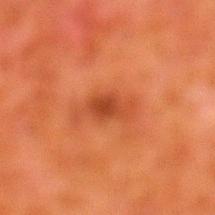Q: Is there a histopathology result?
A: catalogued during a skin exam; not biopsied
Q: Illumination type?
A: cross-polarized illumination
Q: What is the anatomic site?
A: the leg
Q: How was this image acquired?
A: 15 mm crop, total-body photography
Q: What did automated image analysis measure?
A: an average lesion color of about L≈37 a*≈28 b*≈34 (CIELAB) and a lesion–skin lightness drop of about 8
Q: Who is the patient?
A: male, aged 78–82